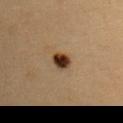No biopsy was performed on this lesion — it was imaged during a full skin examination and was not determined to be concerning. An algorithmic analysis of the crop reported a lesion-detection confidence of about 100/100. A female patient aged approximately 30. Imaged with cross-polarized lighting. The lesion is on the left upper arm. Cropped from a whole-body photographic skin survey; the tile spans about 15 mm.